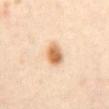Case summary:
- follow-up — total-body-photography surveillance lesion; no biopsy
- imaging modality — total-body-photography crop, ~15 mm field of view
- subject — female, in their 40s
- lesion diameter — ≈3 mm
- anatomic site — the abdomen
- tile lighting — cross-polarized illumination
- automated metrics — an average lesion color of about L≈69 a*≈21 b*≈39 (CIELAB), a lesion–skin lightness drop of about 15, and a normalized border contrast of about 9.5; a border-irregularity rating of about 2/10 and a color-variation rating of about 5/10; a nevus-likeness score of about 100/100 and a detector confidence of about 100 out of 100 that the crop contains a lesion